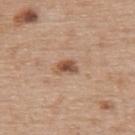workup: catalogued during a skin exam; not biopsied
imaging modality: 15 mm crop, total-body photography
patient: female, aged around 65
anatomic site: the upper back